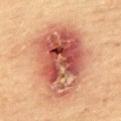{"biopsy_status": "not biopsied; imaged during a skin examination", "lighting": "cross-polarized", "image": {"source": "total-body photography crop", "field_of_view_mm": 15}, "site": "upper back", "automated_metrics": {"cielab_L": 51, "cielab_a": 26, "cielab_b": 30, "vs_skin_darker_L": 14.0, "vs_skin_contrast_norm": 10.0, "color_variation_0_10": 10.0, "peripheral_color_asymmetry": 3.5, "nevus_likeness_0_100": 0, "lesion_detection_confidence_0_100": 100}, "patient": {"sex": "male", "age_approx": 65}}